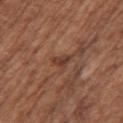Notes:
- workup — catalogued during a skin exam; not biopsied
- patient — female, aged 73 to 77
- automated metrics — a lesion–skin lightness drop of about 8; a color-variation rating of about 1/10 and peripheral color asymmetry of about 0.5; lesion-presence confidence of about 75/100
- lighting — white-light illumination
- image source — ~15 mm tile from a whole-body skin photo
- location — the left upper arm
- size — about 2.5 mm